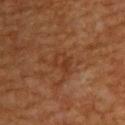Captured during whole-body skin photography for melanoma surveillance; the lesion was not biopsied. The subject is a male aged 58 to 62. A lesion tile, about 15 mm wide, cut from a 3D total-body photograph. The lesion-visualizer software estimated a mean CIELAB color near L≈33 a*≈22 b*≈30, a lesion–skin lightness drop of about 5, and a lesion-to-skin contrast of about 5 (normalized; higher = more distinct). The recorded lesion diameter is about 3 mm. This is a cross-polarized tile. From the upper back.